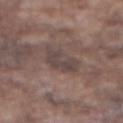Recorded during total-body skin imaging; not selected for excision or biopsy.
About 4.5 mm across.
The lesion is located on the front of the torso.
Captured under white-light illumination.
A 15 mm close-up extracted from a 3D total-body photography capture.
Automated image analysis of the tile measured a footprint of about 7 mm² and an outline eccentricity of about 0.85 (0 = round, 1 = elongated). And it measured a border-irregularity rating of about 5.5/10 and a peripheral color-asymmetry measure near 0.5.
A male subject, in their mid- to late 70s.